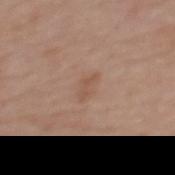Q: Was this lesion biopsied?
A: imaged on a skin check; not biopsied
Q: Lesion location?
A: the upper back
Q: How large is the lesion?
A: ≈3 mm
Q: How was the tile lit?
A: white-light
Q: How was this image acquired?
A: total-body-photography crop, ~15 mm field of view
Q: Patient demographics?
A: female, roughly 60 years of age